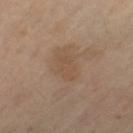biopsy_status: not biopsied; imaged during a skin examination
patient:
  sex: female
  age_approx: 65
lesion_size:
  long_diameter_mm_approx: 3.5
image:
  source: total-body photography crop
  field_of_view_mm: 15
site: left thigh